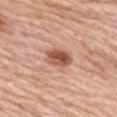Imaged during a routine full-body skin examination; the lesion was not biopsied and no histopathology is available. This image is a 15 mm lesion crop taken from a total-body photograph. Automated image analysis of the tile measured peripheral color asymmetry of about 2.5. A female patient, aged around 65. The lesion is on the upper back. Imaged with white-light lighting. The lesion's longest dimension is about 3.5 mm.